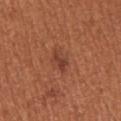Approximately 3 mm at its widest.
A close-up tile cropped from a whole-body skin photograph, about 15 mm across.
A female subject approximately 55 years of age.
This is a white-light tile.
The lesion is located on the left upper arm.